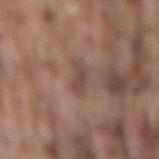The lesion was photographed on a routine skin check and not biopsied; there is no pathology result. The tile uses white-light illumination. A roughly 15 mm field-of-view crop from a total-body skin photograph. The lesion is located on the mid back. Automated tile analysis of the lesion measured an area of roughly 3 mm² and a symmetry-axis asymmetry near 0.35. The software also gave border irregularity of about 3.5 on a 0–10 scale, internal color variation of about 0 on a 0–10 scale, and peripheral color asymmetry of about 0. Longest diameter approximately 3 mm. A male patient in their mid- to late 70s.